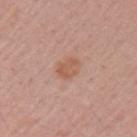biopsy status — catalogued during a skin exam; not biopsied | size — ~3 mm (longest diameter) | automated lesion analysis — an area of roughly 5 mm², an eccentricity of roughly 0.75, and a shape-asymmetry score of about 0.25 (0 = symmetric) | imaging modality — 15 mm crop, total-body photography | site — the left upper arm | lighting — white-light illumination | patient — female, aged around 55.